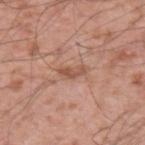Imaged during a routine full-body skin examination; the lesion was not biopsied and no histopathology is available. The tile uses white-light illumination. A 15 mm close-up tile from a total-body photography series done for melanoma screening. Automated image analysis of the tile measured an eccentricity of roughly 0.9 and two-axis asymmetry of about 0.5. It also reported an average lesion color of about L≈52 a*≈22 b*≈30 (CIELAB), about 10 CIELAB-L* units darker than the surrounding skin, and a normalized lesion–skin contrast near 7. The software also gave a border-irregularity rating of about 5/10, a within-lesion color-variation index near 0/10, and peripheral color asymmetry of about 0. And it measured a lesion-detection confidence of about 100/100. About 3 mm across. The lesion is located on the left forearm. The subject is a male aged 38 to 42.